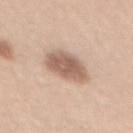Q: Was a biopsy performed?
A: total-body-photography surveillance lesion; no biopsy
Q: Illumination type?
A: white-light
Q: What kind of image is this?
A: 15 mm crop, total-body photography
Q: Who is the patient?
A: female, aged 43 to 47
Q: Automated lesion metrics?
A: a lesion color around L≈60 a*≈18 b*≈27 in CIELAB, roughly 14 lightness units darker than nearby skin, and a normalized lesion–skin contrast near 8.5; border irregularity of about 2 on a 0–10 scale, a color-variation rating of about 3/10, and radial color variation of about 1
Q: What is the anatomic site?
A: the front of the torso